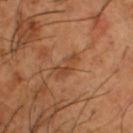Recorded during total-body skin imaging; not selected for excision or biopsy. Automated image analysis of the tile measured border irregularity of about 5 on a 0–10 scale, internal color variation of about 1 on a 0–10 scale, and radial color variation of about 0. A 15 mm crop from a total-body photograph taken for skin-cancer surveillance. Measured at roughly 3.5 mm in maximum diameter. A male patient, in their mid- to late 60s. Captured under cross-polarized illumination. On the left thigh.